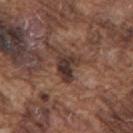| feature | finding |
|---|---|
| image | ~15 mm tile from a whole-body skin photo |
| patient | male, aged 73 to 77 |
| anatomic site | the upper back |
| lesion diameter | about 3 mm |